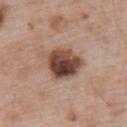Impression: The lesion was photographed on a routine skin check and not biopsied; there is no pathology result. Background: Imaged with white-light lighting. A male subject, about 55 years old. A lesion tile, about 15 mm wide, cut from a 3D total-body photograph. Located on the upper back.